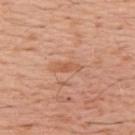workup = catalogued during a skin exam; not biopsied
patient = male, about 70 years old
location = the upper back
image source = ~15 mm tile from a whole-body skin photo